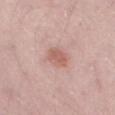Captured during whole-body skin photography for melanoma surveillance; the lesion was not biopsied. A male patient roughly 50 years of age. This image is a 15 mm lesion crop taken from a total-body photograph. Located on the left thigh. The lesion-visualizer software estimated an area of roughly 5 mm², an outline eccentricity of about 0.65 (0 = round, 1 = elongated), and two-axis asymmetry of about 0.2. And it measured an average lesion color of about L≈60 a*≈22 b*≈26 (CIELAB), about 9 CIELAB-L* units darker than the surrounding skin, and a normalized border contrast of about 6.5. And it measured internal color variation of about 1.5 on a 0–10 scale and peripheral color asymmetry of about 0.5. It also reported an automated nevus-likeness rating near 45 out of 100 and a lesion-detection confidence of about 100/100. Measured at roughly 3 mm in maximum diameter.